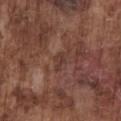The lesion was tiled from a total-body skin photograph and was not biopsied.
Automated image analysis of the tile measured a footprint of about 3 mm², an outline eccentricity of about 0.8 (0 = round, 1 = elongated), and two-axis asymmetry of about 0.4. The software also gave a lesion color around L≈37 a*≈20 b*≈23 in CIELAB, a lesion–skin lightness drop of about 6, and a normalized border contrast of about 5. And it measured an automated nevus-likeness rating near 0 out of 100.
Captured under white-light illumination.
Measured at roughly 2.5 mm in maximum diameter.
A close-up tile cropped from a whole-body skin photograph, about 15 mm across.
From the chest.
A male subject approximately 75 years of age.